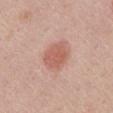• follow-up — catalogued during a skin exam; not biopsied
• lighting — white-light illumination
• site — the chest
• patient — male, aged around 60
• automated metrics — an area of roughly 11 mm², an eccentricity of roughly 0.5, and two-axis asymmetry of about 0.15
• lesion size — ~4 mm (longest diameter)
• image — 15 mm crop, total-body photography